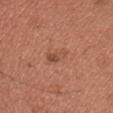follow-up=total-body-photography surveillance lesion; no biopsy | subject=male, in their mid- to late 30s | anatomic site=the chest | image source=~15 mm tile from a whole-body skin photo | diameter=about 4 mm | illumination=white-light illumination.